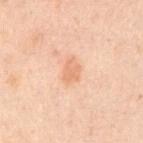{
  "biopsy_status": "not biopsied; imaged during a skin examination",
  "site": "left upper arm",
  "patient": {
    "sex": "male",
    "age_approx": 50
  },
  "image": {
    "source": "total-body photography crop",
    "field_of_view_mm": 15
  }
}